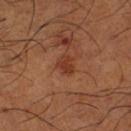Case summary:
* follow-up · catalogued during a skin exam; not biopsied
* patient · male, aged around 65
* acquisition · ~15 mm tile from a whole-body skin photo
* lesion size · ≈2.5 mm
* site · the right thigh
* image-analysis metrics · a shape eccentricity near 0.65 and two-axis asymmetry of about 0.2; a lesion color around L≈37 a*≈26 b*≈32 in CIELAB, about 8 CIELAB-L* units darker than the surrounding skin, and a lesion-to-skin contrast of about 7 (normalized; higher = more distinct); an automated nevus-likeness rating near 25 out of 100 and lesion-presence confidence of about 100/100
* illumination · cross-polarized illumination